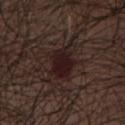biopsy status: total-body-photography surveillance lesion; no biopsy
subject: male, approximately 75 years of age
image-analysis metrics: an area of roughly 12 mm², an outline eccentricity of about 0.75 (0 = round, 1 = elongated), and a symmetry-axis asymmetry near 0.35; a border-irregularity index near 4.5/10, internal color variation of about 5 on a 0–10 scale, and peripheral color asymmetry of about 2
anatomic site: the abdomen
image: total-body-photography crop, ~15 mm field of view
lesion size: about 5 mm
lighting: white-light